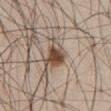notes — no biopsy performed (imaged during a skin exam); tile lighting — white-light illumination; acquisition — ~15 mm tile from a whole-body skin photo; subject — male, in their 60s; location — the abdomen; lesion size — ~4 mm (longest diameter).